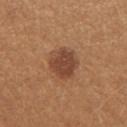Findings:
* follow-up · imaged on a skin check; not biopsied
* anatomic site · the left forearm
* imaging modality · ~15 mm crop, total-body skin-cancer survey
* size · ≈4.5 mm
* patient · female, aged 23 to 27
* TBP lesion metrics · a border-irregularity rating of about 2/10, internal color variation of about 3.5 on a 0–10 scale, and peripheral color asymmetry of about 1
* tile lighting · white-light